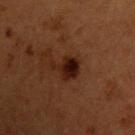Findings:
* biopsy status — total-body-photography surveillance lesion; no biopsy
* lighting — cross-polarized
* image — ~15 mm crop, total-body skin-cancer survey
* anatomic site — the chest
* patient — male, aged around 50
* lesion diameter — ~4.5 mm (longest diameter)